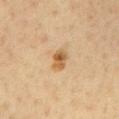The lesion was tiled from a total-body skin photograph and was not biopsied.
The patient is a male approximately 60 years of age.
The lesion is located on the chest.
The total-body-photography lesion software estimated a footprint of about 4.5 mm², an eccentricity of roughly 0.8, and two-axis asymmetry of about 0.25. The analysis additionally found a border-irregularity index near 2/10, a within-lesion color-variation index near 5.5/10, and radial color variation of about 2. It also reported a nevus-likeness score of about 85/100 and a lesion-detection confidence of about 100/100.
This is a cross-polarized tile.
A 15 mm close-up tile from a total-body photography series done for melanoma screening.